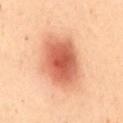| feature | finding |
|---|---|
| image source | total-body-photography crop, ~15 mm field of view |
| subject | female, aged around 50 |
| lesion diameter | about 6.5 mm |
| site | the mid back |